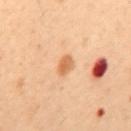{"biopsy_status": "not biopsied; imaged during a skin examination", "lighting": "cross-polarized", "automated_metrics": {"vs_skin_darker_L": 10.0, "vs_skin_contrast_norm": 7.5, "border_irregularity_0_10": 1.5, "color_variation_0_10": 2.5, "peripheral_color_asymmetry": 1.0}, "patient": {"sex": "female", "age_approx": 50}, "lesion_size": {"long_diameter_mm_approx": 2.5}, "image": {"source": "total-body photography crop", "field_of_view_mm": 15}, "site": "back"}